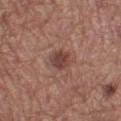No biopsy was performed on this lesion — it was imaged during a full skin examination and was not determined to be concerning.
Automated tile analysis of the lesion measured a footprint of about 5.5 mm², a shape eccentricity near 0.7, and a shape-asymmetry score of about 0.25 (0 = symmetric). The analysis additionally found a lesion-detection confidence of about 100/100.
Captured under white-light illumination.
A region of skin cropped from a whole-body photographic capture, roughly 15 mm wide.
A male subject, in their mid-60s.
The recorded lesion diameter is about 3 mm.
Located on the left lower leg.